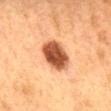Q: Was a biopsy performed?
A: total-body-photography surveillance lesion; no biopsy
Q: Lesion location?
A: the mid back
Q: How was this image acquired?
A: ~15 mm tile from a whole-body skin photo
Q: What is the lesion's diameter?
A: about 4 mm
Q: What did automated image analysis measure?
A: a lesion–skin lightness drop of about 18 and a normalized border contrast of about 13; a border-irregularity rating of about 1/10, a color-variation rating of about 4/10, and peripheral color asymmetry of about 1; a classifier nevus-likeness of about 95/100
Q: Illumination type?
A: cross-polarized illumination
Q: What are the patient's age and sex?
A: male, approximately 75 years of age